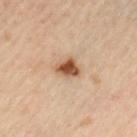{"biopsy_status": "not biopsied; imaged during a skin examination", "lighting": "cross-polarized", "lesion_size": {"long_diameter_mm_approx": 3.0}, "site": "left thigh", "automated_metrics": {"area_mm2_approx": 5.5, "eccentricity": 0.7, "shape_asymmetry": 0.25, "cielab_L": 54, "cielab_a": 21, "cielab_b": 34, "vs_skin_darker_L": 16.0, "lesion_detection_confidence_0_100": 100}, "image": {"source": "total-body photography crop", "field_of_view_mm": 15}, "patient": {"sex": "male", "age_approx": 65}}